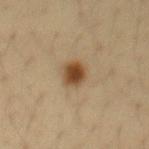Case summary:
- follow-up — catalogued during a skin exam; not biopsied
- image — ~15 mm tile from a whole-body skin photo
- site — the abdomen
- patient — male, aged around 35
- image-analysis metrics — a lesion color around L≈42 a*≈16 b*≈32 in CIELAB and roughly 13 lightness units darker than nearby skin; a border-irregularity rating of about 2/10, internal color variation of about 4 on a 0–10 scale, and radial color variation of about 1; a detector confidence of about 100 out of 100 that the crop contains a lesion
- lighting — cross-polarized